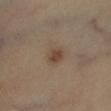Assessment:
Part of a total-body skin-imaging series; this lesion was reviewed on a skin check and was not flagged for biopsy.
Clinical summary:
Captured under cross-polarized illumination. On the right lower leg. The patient is a male approximately 55 years of age. A 15 mm close-up extracted from a 3D total-body photography capture. About 2.5 mm across. Automated tile analysis of the lesion measured a lesion–skin lightness drop of about 8 and a normalized border contrast of about 7.5. The analysis additionally found a classifier nevus-likeness of about 85/100 and a detector confidence of about 100 out of 100 that the crop contains a lesion.